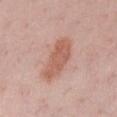Impression:
Captured during whole-body skin photography for melanoma surveillance; the lesion was not biopsied.
Background:
The lesion's longest dimension is about 6 mm. A 15 mm close-up tile from a total-body photography series done for melanoma screening. On the chest. A male subject, aged 58 to 62. Automated tile analysis of the lesion measured a footprint of about 13 mm², a shape eccentricity near 0.9, and a shape-asymmetry score of about 0.25 (0 = symmetric). And it measured a lesion color around L≈59 a*≈23 b*≈28 in CIELAB and roughly 10 lightness units darker than nearby skin. The analysis additionally found border irregularity of about 3 on a 0–10 scale, internal color variation of about 2.5 on a 0–10 scale, and a peripheral color-asymmetry measure near 1.